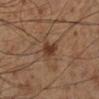Image and clinical context: A region of skin cropped from a whole-body photographic capture, roughly 15 mm wide. A male subject about 60 years old. From the right lower leg.